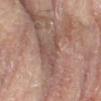| feature | finding |
|---|---|
| follow-up | imaged on a skin check; not biopsied |
| image | ~15 mm tile from a whole-body skin photo |
| subject | female, about 80 years old |
| site | the left leg |